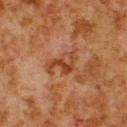Imaged during a routine full-body skin examination; the lesion was not biopsied and no histopathology is available. On the upper back. Cropped from a total-body skin-imaging series; the visible field is about 15 mm. The patient is a male aged around 80.